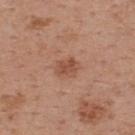patient:
  sex: male
  age_approx: 55
automated_metrics:
  area_mm2_approx: 5.0
  eccentricity: 0.6
  shape_asymmetry: 0.25
  border_irregularity_0_10: 2.0
  color_variation_0_10: 2.5
  peripheral_color_asymmetry: 1.0
  lesion_detection_confidence_0_100: 100
lesion_size:
  long_diameter_mm_approx: 2.5
site: upper back
image:
  source: total-body photography crop
  field_of_view_mm: 15
lighting: white-light